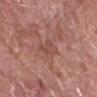This lesion was catalogued during total-body skin photography and was not selected for biopsy. This is a white-light tile. The lesion-visualizer software estimated an outline eccentricity of about 0.85 (0 = round, 1 = elongated) and a symmetry-axis asymmetry near 0.4. And it measured an average lesion color of about L≈48 a*≈23 b*≈25 (CIELAB) and about 6 CIELAB-L* units darker than the surrounding skin. The software also gave internal color variation of about 1.5 on a 0–10 scale and a peripheral color-asymmetry measure near 0.5. The software also gave a classifier nevus-likeness of about 0/100 and a lesion-detection confidence of about 95/100. A roughly 15 mm field-of-view crop from a total-body skin photograph. The lesion is located on the right forearm. About 3.5 mm across. A male subject, aged 78–82.